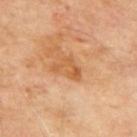Part of a total-body skin-imaging series; this lesion was reviewed on a skin check and was not flagged for biopsy. On the mid back. Automated tile analysis of the lesion measured a shape eccentricity near 0.75. The analysis additionally found an average lesion color of about L≈57 a*≈24 b*≈41 (CIELAB), a lesion–skin lightness drop of about 8, and a lesion-to-skin contrast of about 6.5 (normalized; higher = more distinct). It also reported a color-variation rating of about 0/10 and peripheral color asymmetry of about 0. The recorded lesion diameter is about 3.5 mm. The patient is a male aged 68 to 72. Captured under cross-polarized illumination. Cropped from a total-body skin-imaging series; the visible field is about 15 mm.